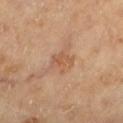follow-up: imaged on a skin check; not biopsied | anatomic site: the left lower leg | patient: female, aged 58–62 | image: total-body-photography crop, ~15 mm field of view.